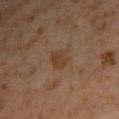The lesion was tiled from a total-body skin photograph and was not biopsied.
The subject is a male aged 58–62.
The lesion is located on the right upper arm.
A close-up tile cropped from a whole-body skin photograph, about 15 mm across.
Measured at roughly 3 mm in maximum diameter.